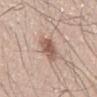Findings:
– follow-up — no biopsy performed (imaged during a skin exam)
– imaging modality — ~15 mm tile from a whole-body skin photo
– diameter — ≈4.5 mm
– patient — male, roughly 45 years of age
– body site — the mid back